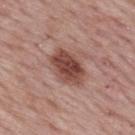Notes:
* follow-up — no biopsy performed (imaged during a skin exam)
* subject — male, in their mid-60s
* illumination — white-light illumination
* imaging modality — ~15 mm tile from a whole-body skin photo
* location — the upper back